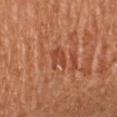workup=catalogued during a skin exam; not biopsied | TBP lesion metrics=a border-irregularity index near 4.5/10 and radial color variation of about 0.5; a classifier nevus-likeness of about 0/100 and lesion-presence confidence of about 80/100 | lighting=cross-polarized | subject=female, aged around 65 | body site=the arm | acquisition=total-body-photography crop, ~15 mm field of view | diameter=~3 mm (longest diameter).